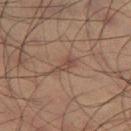Measured at roughly 3 mm in maximum diameter.
This is a cross-polarized tile.
A male patient, roughly 60 years of age.
This image is a 15 mm lesion crop taken from a total-body photograph.
Automated image analysis of the tile measured an average lesion color of about L≈39 a*≈16 b*≈22 (CIELAB), a lesion–skin lightness drop of about 7, and a normalized border contrast of about 6.5. And it measured border irregularity of about 5 on a 0–10 scale, a within-lesion color-variation index near 1.5/10, and a peripheral color-asymmetry measure near 0.5. The analysis additionally found a classifier nevus-likeness of about 5/100.